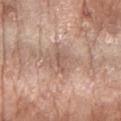Q: Was this lesion biopsied?
A: catalogued during a skin exam; not biopsied
Q: What is the anatomic site?
A: the arm
Q: What kind of image is this?
A: ~15 mm crop, total-body skin-cancer survey
Q: Patient demographics?
A: female, about 75 years old
Q: What did automated image analysis measure?
A: a classifier nevus-likeness of about 0/100 and lesion-presence confidence of about 70/100